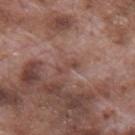<tbp_lesion>
<biopsy_status>not biopsied; imaged during a skin examination</biopsy_status>
<automated_metrics>
  <cielab_L>45</cielab_L>
  <cielab_a>21</cielab_a>
  <cielab_b>24</cielab_b>
  <vs_skin_darker_L>7.0</vs_skin_darker_L>
  <vs_skin_contrast_norm>5.5</vs_skin_contrast_norm>
  <border_irregularity_0_10>5.0</border_irregularity_0_10>
  <color_variation_0_10>0.0</color_variation_0_10>
  <peripheral_color_asymmetry>0.0</peripheral_color_asymmetry>
</automated_metrics>
<site>mid back</site>
<image>
  <source>total-body photography crop</source>
  <field_of_view_mm>15</field_of_view_mm>
</image>
<patient>
  <sex>male</sex>
  <age_approx>70</age_approx>
</patient>
<lesion_size>
  <long_diameter_mm_approx>3.0</long_diameter_mm_approx>
</lesion_size>
</tbp_lesion>